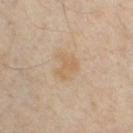Assessment:
The lesion was photographed on a routine skin check and not biopsied; there is no pathology result.
Context:
The lesion is located on the chest. The patient is a male roughly 35 years of age. A region of skin cropped from a whole-body photographic capture, roughly 15 mm wide.